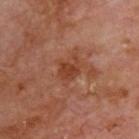Imaged during a routine full-body skin examination; the lesion was not biopsied and no histopathology is available.
The patient is a male aged 68–72.
On the upper back.
A 15 mm crop from a total-body photograph taken for skin-cancer surveillance.